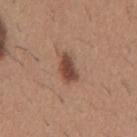Clinical impression: The lesion was photographed on a routine skin check and not biopsied; there is no pathology result.